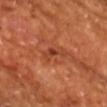Q: Is there a histopathology result?
A: no biopsy performed (imaged during a skin exam)
Q: Lesion size?
A: about 3 mm
Q: Patient demographics?
A: male, aged 63–67
Q: What kind of image is this?
A: ~15 mm crop, total-body skin-cancer survey
Q: What lighting was used for the tile?
A: cross-polarized